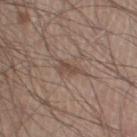The lesion was photographed on a routine skin check and not biopsied; there is no pathology result.
A male subject roughly 30 years of age.
About 2.5 mm across.
A 15 mm crop from a total-body photograph taken for skin-cancer surveillance.
On the right thigh.
Automated tile analysis of the lesion measured an average lesion color of about L≈47 a*≈16 b*≈24 (CIELAB), a lesion–skin lightness drop of about 8, and a normalized lesion–skin contrast near 6.5. It also reported a color-variation rating of about 1/10 and a peripheral color-asymmetry measure near 0.5. The analysis additionally found an automated nevus-likeness rating near 0 out of 100 and lesion-presence confidence of about 95/100.